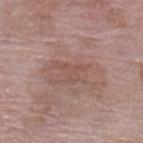Impression:
Imaged during a routine full-body skin examination; the lesion was not biopsied and no histopathology is available.
Image and clinical context:
The tile uses white-light illumination. Approximately 4 mm at its widest. The total-body-photography lesion software estimated a lesion area of about 5.5 mm², an eccentricity of roughly 0.8, and a shape-asymmetry score of about 0.65 (0 = symmetric). The software also gave an average lesion color of about L≈51 a*≈20 b*≈24 (CIELAB), roughly 6 lightness units darker than nearby skin, and a lesion-to-skin contrast of about 4.5 (normalized; higher = more distinct). And it measured border irregularity of about 7 on a 0–10 scale, a within-lesion color-variation index near 1/10, and radial color variation of about 0.5. It also reported a classifier nevus-likeness of about 0/100 and a lesion-detection confidence of about 95/100. Located on the left lower leg. A 15 mm crop from a total-body photograph taken for skin-cancer surveillance. The subject is a female aged 68 to 72.